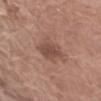Clinical impression: Imaged during a routine full-body skin examination; the lesion was not biopsied and no histopathology is available. Image and clinical context: A female subject, in their mid- to late 70s. The lesion is located on the left upper arm. A lesion tile, about 15 mm wide, cut from a 3D total-body photograph. Measured at roughly 3.5 mm in maximum diameter.